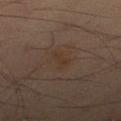Findings:
* TBP lesion metrics: a footprint of about 4 mm²; a mean CIELAB color near L≈34 a*≈14 b*≈24; a border-irregularity rating of about 4.5/10, internal color variation of about 1 on a 0–10 scale, and radial color variation of about 0.5; an automated nevus-likeness rating near 0 out of 100 and a detector confidence of about 100 out of 100 that the crop contains a lesion
* subject: male, aged approximately 40
* tile lighting: cross-polarized illumination
* image source: ~15 mm tile from a whole-body skin photo
* anatomic site: the right thigh